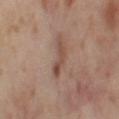{"biopsy_status": "not biopsied; imaged during a skin examination", "patient": {"sex": "female", "age_approx": 55}, "lesion_size": {"long_diameter_mm_approx": 4.0}, "lighting": "cross-polarized", "image": {"source": "total-body photography crop", "field_of_view_mm": 15}, "automated_metrics": {"area_mm2_approx": 4.5, "eccentricity": 0.95, "color_variation_0_10": 0.5, "peripheral_color_asymmetry": 0.0, "nevus_likeness_0_100": 0, "lesion_detection_confidence_0_100": 100}, "site": "right thigh"}